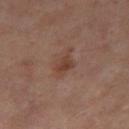The lesion was tiled from a total-body skin photograph and was not biopsied.
Approximately 2.5 mm at its widest.
Located on the left thigh.
A female patient roughly 50 years of age.
An algorithmic analysis of the crop reported a lesion area of about 3.5 mm², a shape eccentricity near 0.75, and a symmetry-axis asymmetry near 0.35. The software also gave an average lesion color of about L≈38 a*≈19 b*≈25 (CIELAB) and about 8 CIELAB-L* units darker than the surrounding skin. And it measured a detector confidence of about 100 out of 100 that the crop contains a lesion.
A lesion tile, about 15 mm wide, cut from a 3D total-body photograph.
Captured under cross-polarized illumination.